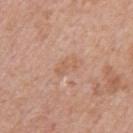Impression:
Imaged during a routine full-body skin examination; the lesion was not biopsied and no histopathology is available.
Clinical summary:
The recorded lesion diameter is about 3 mm. A male subject aged approximately 60. A region of skin cropped from a whole-body photographic capture, roughly 15 mm wide. Imaged with white-light lighting. From the right upper arm.